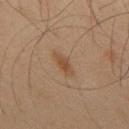An algorithmic analysis of the crop reported a footprint of about 3.5 mm², an outline eccentricity of about 0.9 (0 = round, 1 = elongated), and a shape-asymmetry score of about 0.25 (0 = symmetric). And it measured a mean CIELAB color near L≈38 a*≈15 b*≈27 and a normalized border contrast of about 7. The software also gave border irregularity of about 3 on a 0–10 scale, a within-lesion color-variation index near 1/10, and a peripheral color-asymmetry measure near 0.
Imaged with cross-polarized lighting.
The lesion is on the upper back.
A 15 mm close-up extracted from a 3D total-body photography capture.
A male patient about 65 years old.
The lesion's longest dimension is about 3 mm.